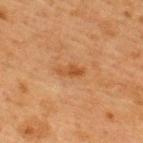  biopsy_status: not biopsied; imaged during a skin examination
  image:
    source: total-body photography crop
    field_of_view_mm: 15
  lesion_size:
    long_diameter_mm_approx: 3.0
  site: upper back
  lighting: cross-polarized
  patient:
    sex: female
    age_approx: 40